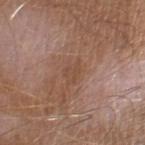Q: Was this lesion biopsied?
A: imaged on a skin check; not biopsied
Q: Where on the body is the lesion?
A: the right thigh
Q: How large is the lesion?
A: ~3 mm (longest diameter)
Q: What did automated image analysis measure?
A: a classifier nevus-likeness of about 0/100 and a detector confidence of about 100 out of 100 that the crop contains a lesion
Q: What are the patient's age and sex?
A: male, in their mid-60s
Q: How was this image acquired?
A: total-body-photography crop, ~15 mm field of view